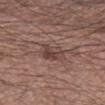Recorded during total-body skin imaging; not selected for excision or biopsy.
Measured at roughly 2.5 mm in maximum diameter.
The tile uses white-light illumination.
A 15 mm close-up extracted from a 3D total-body photography capture.
The patient is a male about 65 years old.
From the left forearm.